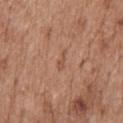Captured under white-light illumination.
A male subject, in their 60s.
A 15 mm crop from a total-body photograph taken for skin-cancer surveillance.
Measured at roughly 2.5 mm in maximum diameter.
An algorithmic analysis of the crop reported roughly 7 lightness units darker than nearby skin. And it measured a border-irregularity index near 5.5/10 and a color-variation rating of about 0/10.
The lesion is located on the mid back.